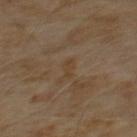The lesion was photographed on a routine skin check and not biopsied; there is no pathology result. A male patient, aged approximately 60. Imaged with cross-polarized lighting. The lesion's longest dimension is about 2.5 mm. A 15 mm crop from a total-body photograph taken for skin-cancer surveillance. Automated tile analysis of the lesion measured an average lesion color of about L≈38 a*≈14 b*≈28 (CIELAB) and roughly 4 lightness units darker than nearby skin. Located on the chest.